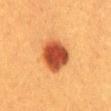Recorded during total-body skin imaging; not selected for excision or biopsy. A roughly 15 mm field-of-view crop from a total-body skin photograph. On the mid back. Approximately 4.5 mm at its widest. A female subject, about 40 years old. The total-body-photography lesion software estimated a lesion area of about 13 mm², an outline eccentricity of about 0.65 (0 = round, 1 = elongated), and a shape-asymmetry score of about 0.15 (0 = symmetric). And it measured a border-irregularity index near 1.5/10, a color-variation rating of about 4/10, and peripheral color asymmetry of about 1. The analysis additionally found lesion-presence confidence of about 100/100.